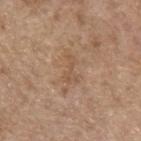Captured under white-light illumination.
Approximately 3.5 mm at its widest.
A close-up tile cropped from a whole-body skin photograph, about 15 mm across.
A female subject roughly 65 years of age.
The lesion is on the arm.